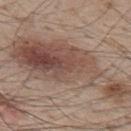Impression:
No biopsy was performed on this lesion — it was imaged during a full skin examination and was not determined to be concerning.
Clinical summary:
A roughly 15 mm field-of-view crop from a total-body skin photograph. From the upper back. A male patient aged 48 to 52. The lesion's longest dimension is about 15 mm. Captured under white-light illumination.